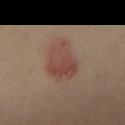Part of a total-body skin-imaging series; this lesion was reviewed on a skin check and was not flagged for biopsy. A 15 mm crop from a total-body photograph taken for skin-cancer surveillance. Imaged with cross-polarized lighting. The lesion is located on the lower back. The patient is a female about 35 years old.